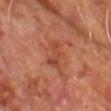Q: Is there a histopathology result?
A: no biopsy performed (imaged during a skin exam)
Q: Where on the body is the lesion?
A: the upper back
Q: Patient demographics?
A: male, approximately 60 years of age
Q: Automated lesion metrics?
A: a footprint of about 6 mm² and a shape-asymmetry score of about 0.4 (0 = symmetric); internal color variation of about 2 on a 0–10 scale and peripheral color asymmetry of about 0.5; an automated nevus-likeness rating near 0 out of 100
Q: How was the tile lit?
A: cross-polarized illumination
Q: How large is the lesion?
A: ~4 mm (longest diameter)
Q: What kind of image is this?
A: ~15 mm crop, total-body skin-cancer survey